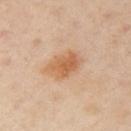workup: imaged on a skin check; not biopsied
anatomic site: the left arm
subject: female, aged 38 to 42
lighting: cross-polarized
image source: total-body-photography crop, ~15 mm field of view